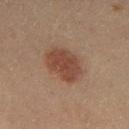Case summary:
* follow-up: imaged on a skin check; not biopsied
* automated lesion analysis: an area of roughly 12 mm², an outline eccentricity of about 0.8 (0 = round, 1 = elongated), and two-axis asymmetry of about 0.2; a border-irregularity index near 2/10, internal color variation of about 2.5 on a 0–10 scale, and radial color variation of about 1; an automated nevus-likeness rating near 100 out of 100 and a lesion-detection confidence of about 100/100
* patient: female, aged around 50
* lighting: cross-polarized
* lesion diameter: ~5 mm (longest diameter)
* body site: the left thigh
* image: 15 mm crop, total-body photography